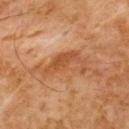| feature | finding |
|---|---|
| follow-up | no biopsy performed (imaged during a skin exam) |
| subject | male, aged 58–62 |
| location | the upper back |
| acquisition | ~15 mm tile from a whole-body skin photo |